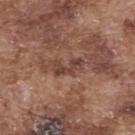  biopsy_status: not biopsied; imaged during a skin examination
  image:
    source: total-body photography crop
    field_of_view_mm: 15
  patient:
    sex: male
    age_approx: 75
  automated_metrics:
    area_mm2_approx: 4.5
    eccentricity: 0.9
    shape_asymmetry: 0.4
    border_irregularity_0_10: 5.5
    color_variation_0_10: 1.0
    peripheral_color_asymmetry: 0.5
  lighting: white-light
  lesion_size:
    long_diameter_mm_approx: 3.5
  site: upper back